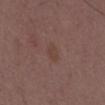Background: A male patient, aged 48–52. About 2.5 mm across. Automated image analysis of the tile measured a footprint of about 3.5 mm², an outline eccentricity of about 0.8 (0 = round, 1 = elongated), and a shape-asymmetry score of about 0.2 (0 = symmetric). And it measured an average lesion color of about L≈41 a*≈18 b*≈22 (CIELAB), about 4 CIELAB-L* units darker than the surrounding skin, and a lesion-to-skin contrast of about 5 (normalized; higher = more distinct). And it measured an automated nevus-likeness rating near 0 out of 100. Cropped from a whole-body photographic skin survey; the tile spans about 15 mm. From the chest. The tile uses white-light illumination.